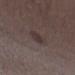• notes — catalogued during a skin exam; not biopsied
• subject — female, about 35 years old
• imaging modality — total-body-photography crop, ~15 mm field of view
• body site — the left lower leg